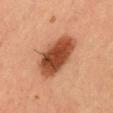Case summary:
– biopsy status: catalogued during a skin exam; not biopsied
– lighting: cross-polarized illumination
– image source: ~15 mm crop, total-body skin-cancer survey
– location: the chest
– lesion diameter: ~7 mm (longest diameter)
– patient: male, roughly 35 years of age